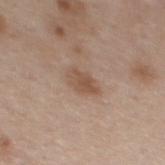Acquisition and patient details:
Imaged with white-light lighting. A female subject, approximately 40 years of age. A lesion tile, about 15 mm wide, cut from a 3D total-body photograph. An algorithmic analysis of the crop reported a footprint of about 5 mm², an outline eccentricity of about 0.8 (0 = round, 1 = elongated), and a symmetry-axis asymmetry near 0.2. The software also gave an average lesion color of about L≈51 a*≈18 b*≈29 (CIELAB), a lesion–skin lightness drop of about 9, and a lesion-to-skin contrast of about 7 (normalized; higher = more distinct). The analysis additionally found an automated nevus-likeness rating near 15 out of 100 and a detector confidence of about 100 out of 100 that the crop contains a lesion. On the back.